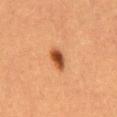Assessment:
The lesion was tiled from a total-body skin photograph and was not biopsied.
Image and clinical context:
The tile uses cross-polarized illumination. Measured at roughly 3 mm in maximum diameter. The lesion is located on the mid back. A roughly 15 mm field-of-view crop from a total-body skin photograph. A female patient in their mid- to late 50s.